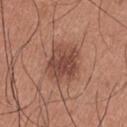Background:
The recorded lesion diameter is about 5 mm. A 15 mm crop from a total-body photograph taken for skin-cancer surveillance. A male subject aged 33–37. Automated image analysis of the tile measured a within-lesion color-variation index near 4/10 and a peripheral color-asymmetry measure near 1.5. The lesion is on the right upper arm. Captured under white-light illumination.